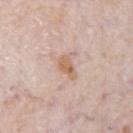Imaged during a routine full-body skin examination; the lesion was not biopsied and no histopathology is available. On the chest. This is a white-light tile. A male patient, aged 78 to 82. A roughly 15 mm field-of-view crop from a total-body skin photograph.